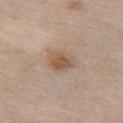Case summary:
– workup: total-body-photography surveillance lesion; no biopsy
– lighting: white-light
– size: about 3.5 mm
– patient: female, aged 63–67
– location: the front of the torso
– acquisition: ~15 mm tile from a whole-body skin photo